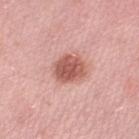Q: What is the imaging modality?
A: ~15 mm tile from a whole-body skin photo
Q: Lesion location?
A: the left thigh
Q: Patient demographics?
A: female, aged approximately 40
Q: How large is the lesion?
A: about 3.5 mm